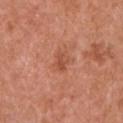Q: Was a biopsy performed?
A: catalogued during a skin exam; not biopsied
Q: Automated lesion metrics?
A: a footprint of about 3.5 mm² and two-axis asymmetry of about 0.3; border irregularity of about 3 on a 0–10 scale and radial color variation of about 1
Q: What is the lesion's diameter?
A: ~2.5 mm (longest diameter)
Q: How was this image acquired?
A: ~15 mm crop, total-body skin-cancer survey
Q: What lighting was used for the tile?
A: white-light illumination
Q: Patient demographics?
A: male, aged around 65
Q: Where on the body is the lesion?
A: the left upper arm